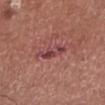Assessment: The lesion was tiled from a total-body skin photograph and was not biopsied.